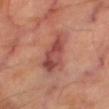Context:
Imaged with cross-polarized lighting. The patient is a male approximately 70 years of age. The lesion is located on the leg. A close-up tile cropped from a whole-body skin photograph, about 15 mm across.